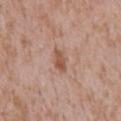Impression:
This lesion was catalogued during total-body skin photography and was not selected for biopsy.
Clinical summary:
Captured under white-light illumination. The lesion is on the mid back. A male subject aged approximately 45. The lesion-visualizer software estimated a shape eccentricity near 0.85 and a shape-asymmetry score of about 0.25 (0 = symmetric). And it measured a mean CIELAB color near L≈53 a*≈21 b*≈29, roughly 10 lightness units darker than nearby skin, and a normalized lesion–skin contrast near 7.5. It also reported border irregularity of about 2.5 on a 0–10 scale, a within-lesion color-variation index near 2/10, and a peripheral color-asymmetry measure near 0.5. The software also gave a classifier nevus-likeness of about 20/100 and a detector confidence of about 100 out of 100 that the crop contains a lesion. A lesion tile, about 15 mm wide, cut from a 3D total-body photograph. Approximately 2.5 mm at its widest.